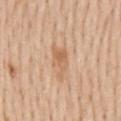Q: Lesion size?
A: about 4 mm
Q: Illumination type?
A: white-light
Q: How was this image acquired?
A: ~15 mm crop, total-body skin-cancer survey
Q: Patient demographics?
A: male, about 60 years old
Q: Where on the body is the lesion?
A: the mid back